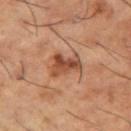<tbp_lesion>
  <biopsy_status>not biopsied; imaged during a skin examination</biopsy_status>
  <image>
    <source>total-body photography crop</source>
    <field_of_view_mm>15</field_of_view_mm>
  </image>
  <lesion_size>
    <long_diameter_mm_approx>4.0</long_diameter_mm_approx>
  </lesion_size>
  <lighting>cross-polarized</lighting>
  <patient>
    <sex>male</sex>
    <age_approx>65</age_approx>
  </patient>
  <site>right thigh</site>
</tbp_lesion>